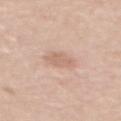Case summary:
* follow-up: total-body-photography surveillance lesion; no biopsy
* patient: male, aged 58–62
* automated metrics: an area of roughly 5 mm², an outline eccentricity of about 0.6 (0 = round, 1 = elongated), and a shape-asymmetry score of about 0.25 (0 = symmetric); about 8 CIELAB-L* units darker than the surrounding skin and a normalized lesion–skin contrast near 5; border irregularity of about 2.5 on a 0–10 scale and internal color variation of about 1.5 on a 0–10 scale
* location: the upper back
* tile lighting: white-light illumination
* lesion diameter: about 3 mm
* image: 15 mm crop, total-body photography